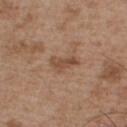Q: Was this lesion biopsied?
A: total-body-photography surveillance lesion; no biopsy
Q: What kind of image is this?
A: ~15 mm tile from a whole-body skin photo
Q: Lesion location?
A: the chest
Q: What did automated image analysis measure?
A: a lesion area of about 4.5 mm², an outline eccentricity of about 0.85 (0 = round, 1 = elongated), and a symmetry-axis asymmetry near 0.4; an average lesion color of about L≈48 a*≈19 b*≈30 (CIELAB), roughly 9 lightness units darker than nearby skin, and a lesion-to-skin contrast of about 7 (normalized; higher = more distinct); a border-irregularity index near 4/10 and radial color variation of about 1
Q: How was the tile lit?
A: white-light illumination
Q: Patient demographics?
A: male, roughly 55 years of age